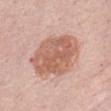Impression: Recorded during total-body skin imaging; not selected for excision or biopsy. Acquisition and patient details: A 15 mm close-up extracted from a 3D total-body photography capture. Located on the abdomen. The lesion-visualizer software estimated a lesion area of about 29 mm², a shape eccentricity near 0.65, and two-axis asymmetry of about 0.1. And it measured an average lesion color of about L≈61 a*≈22 b*≈29 (CIELAB), a lesion–skin lightness drop of about 12, and a normalized lesion–skin contrast near 8. A female subject about 65 years old.